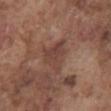<lesion>
<site>abdomen</site>
<lesion_size>
  <long_diameter_mm_approx>4.5</long_diameter_mm_approx>
</lesion_size>
<image>
  <source>total-body photography crop</source>
  <field_of_view_mm>15</field_of_view_mm>
</image>
<patient>
  <sex>male</sex>
  <age_approx>75</age_approx>
</patient>
</lesion>